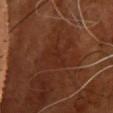This lesion was catalogued during total-body skin photography and was not selected for biopsy. The lesion is on the chest. Cropped from a whole-body photographic skin survey; the tile spans about 15 mm. A male patient approximately 65 years of age. An algorithmic analysis of the crop reported a border-irregularity rating of about 5.5/10 and a peripheral color-asymmetry measure near 0.5. The analysis additionally found a nevus-likeness score of about 10/100 and a lesion-detection confidence of about 85/100.